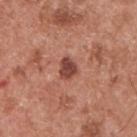Imaged during a routine full-body skin examination; the lesion was not biopsied and no histopathology is available. This is a white-light tile. A male patient aged approximately 55. From the upper back. Cropped from a total-body skin-imaging series; the visible field is about 15 mm. About 2.5 mm across.